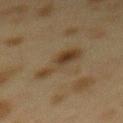Q: Was a biopsy performed?
A: imaged on a skin check; not biopsied
Q: How large is the lesion?
A: ~6 mm (longest diameter)
Q: Automated lesion metrics?
A: a shape eccentricity near 0.95; a lesion color around L≈32 a*≈11 b*≈26 in CIELAB, a lesion–skin lightness drop of about 7, and a lesion-to-skin contrast of about 7.5 (normalized; higher = more distinct); a nevus-likeness score of about 75/100
Q: Who is the patient?
A: female, in their 40s
Q: Lesion location?
A: the mid back
Q: What lighting was used for the tile?
A: cross-polarized illumination
Q: How was this image acquired?
A: total-body-photography crop, ~15 mm field of view